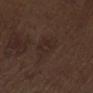Recorded during total-body skin imaging; not selected for excision or biopsy.
Located on the abdomen.
The tile uses white-light illumination.
A male patient, roughly 70 years of age.
About 4 mm across.
A region of skin cropped from a whole-body photographic capture, roughly 15 mm wide.